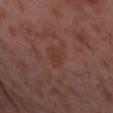No biopsy was performed on this lesion — it was imaged during a full skin examination and was not determined to be concerning. The lesion is on the leg. An algorithmic analysis of the crop reported an area of roughly 5 mm², an outline eccentricity of about 0.85 (0 = round, 1 = elongated), and a shape-asymmetry score of about 0.25 (0 = symmetric). A roughly 15 mm field-of-view crop from a total-body skin photograph. A female patient, in their mid- to late 50s. Approximately 3.5 mm at its widest. Imaged with cross-polarized lighting.